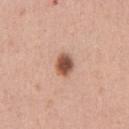biopsy_status: not biopsied; imaged during a skin examination
patient:
  sex: male
  age_approx: 55
lesion_size:
  long_diameter_mm_approx: 2.5
site: abdomen
image:
  source: total-body photography crop
  field_of_view_mm: 15
automated_metrics:
  cielab_L: 53
  cielab_a: 23
  cielab_b: 29
  vs_skin_darker_L: 17.0
  vs_skin_contrast_norm: 11.0
  border_irregularity_0_10: 1.0
  color_variation_0_10: 4.5
  peripheral_color_asymmetry: 1.0
  nevus_likeness_0_100: 100
  lesion_detection_confidence_0_100: 100